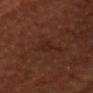{
  "biopsy_status": "not biopsied; imaged during a skin examination",
  "patient": {
    "sex": "male",
    "age_approx": 60
  },
  "site": "head or neck",
  "image": {
    "source": "total-body photography crop",
    "field_of_view_mm": 15
  }
}